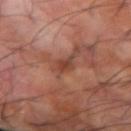lighting: cross-polarized illumination | automated lesion analysis: a footprint of about 3.5 mm², an outline eccentricity of about 0.85 (0 = round, 1 = elongated), and two-axis asymmetry of about 0.35; border irregularity of about 3 on a 0–10 scale, a within-lesion color-variation index near 2.5/10, and peripheral color asymmetry of about 1; a nevus-likeness score of about 0/100 and lesion-presence confidence of about 60/100 | lesion diameter: ≈3 mm | imaging modality: total-body-photography crop, ~15 mm field of view | patient: male, roughly 70 years of age | location: the left forearm.